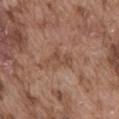The total-body-photography lesion software estimated an area of roughly 4.5 mm², an eccentricity of roughly 0.9, and a symmetry-axis asymmetry near 0.5. The analysis additionally found border irregularity of about 6 on a 0–10 scale, a within-lesion color-variation index near 0.5/10, and peripheral color asymmetry of about 0.
Cropped from a total-body skin-imaging series; the visible field is about 15 mm.
This is a white-light tile.
The subject is a male about 75 years old.
On the abdomen.
About 3.5 mm across.